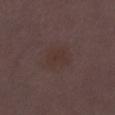Part of a total-body skin-imaging series; this lesion was reviewed on a skin check and was not flagged for biopsy.
A female subject aged 28–32.
Approximately 3.5 mm at its widest.
A close-up tile cropped from a whole-body skin photograph, about 15 mm across.
The lesion is on the leg.
Captured under white-light illumination.